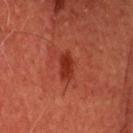image source = ~15 mm tile from a whole-body skin photo | patient = male, aged around 60 | body site = the head or neck | TBP lesion metrics = a lesion–skin lightness drop of about 8; a border-irregularity rating of about 2/10, a within-lesion color-variation index near 1.5/10, and a peripheral color-asymmetry measure near 0.5; a classifier nevus-likeness of about 85/100 and a detector confidence of about 100 out of 100 that the crop contains a lesion | size = ≈3.5 mm.